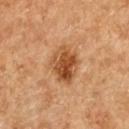Recorded during total-body skin imaging; not selected for excision or biopsy. The lesion-visualizer software estimated an outline eccentricity of about 0.65 (0 = round, 1 = elongated) and a shape-asymmetry score of about 0.15 (0 = symmetric). It also reported a mean CIELAB color near L≈47 a*≈23 b*≈37, a lesion–skin lightness drop of about 12, and a normalized lesion–skin contrast near 9. The analysis additionally found a border-irregularity rating of about 1.5/10, a within-lesion color-variation index near 7/10, and peripheral color asymmetry of about 2.5. This image is a 15 mm lesion crop taken from a total-body photograph. Approximately 4.5 mm at its widest. Imaged with cross-polarized lighting. The patient is a female in their 60s. The lesion is on the upper back.